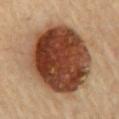Captured during whole-body skin photography for melanoma surveillance; the lesion was not biopsied. A 15 mm close-up tile from a total-body photography series done for melanoma screening. About 9.5 mm across. From the chest. The subject is a female about 70 years old.